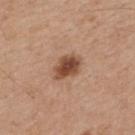Captured during whole-body skin photography for melanoma surveillance; the lesion was not biopsied.
The lesion is located on the back.
Cropped from a whole-body photographic skin survey; the tile spans about 15 mm.
A male subject approximately 65 years of age.
About 3.5 mm across.